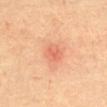Q: Is there a histopathology result?
A: imaged on a skin check; not biopsied
Q: What is the imaging modality?
A: total-body-photography crop, ~15 mm field of view
Q: Who is the patient?
A: male, approximately 70 years of age
Q: Where on the body is the lesion?
A: the chest